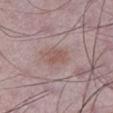Q: Was this lesion biopsied?
A: no biopsy performed (imaged during a skin exam)
Q: What is the lesion's diameter?
A: ~3 mm (longest diameter)
Q: What is the anatomic site?
A: the leg
Q: Who is the patient?
A: male, aged 48 to 52
Q: What kind of image is this?
A: ~15 mm crop, total-body skin-cancer survey
Q: Illumination type?
A: white-light